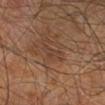Assessment:
Captured during whole-body skin photography for melanoma surveillance; the lesion was not biopsied.
Context:
A lesion tile, about 15 mm wide, cut from a 3D total-body photograph. A male subject about 60 years old. On the right leg.